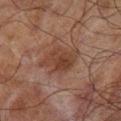Impression:
This lesion was catalogued during total-body skin photography and was not selected for biopsy.
Context:
Cropped from a whole-body photographic skin survey; the tile spans about 15 mm. The total-body-photography lesion software estimated an area of roughly 12 mm², an eccentricity of roughly 0.65, and a shape-asymmetry score of about 0.25 (0 = symmetric). And it measured a mean CIELAB color near L≈33 a*≈17 b*≈23, about 7 CIELAB-L* units darker than the surrounding skin, and a normalized lesion–skin contrast near 7. It also reported a border-irregularity rating of about 3/10, a within-lesion color-variation index near 3.5/10, and radial color variation of about 1. The analysis additionally found a nevus-likeness score of about 10/100 and lesion-presence confidence of about 100/100. The tile uses cross-polarized illumination. Located on the leg. Measured at roughly 5 mm in maximum diameter. A male patient aged 68 to 72.